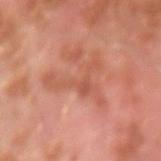biopsy status: catalogued during a skin exam; not biopsied.